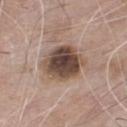The lesion was photographed on a routine skin check and not biopsied; there is no pathology result. Measured at roughly 5 mm in maximum diameter. The patient is a male in their mid- to late 70s. The tile uses white-light illumination. Automated tile analysis of the lesion measured an area of roughly 17 mm², an eccentricity of roughly 0.45, and a symmetry-axis asymmetry near 0.15. The analysis additionally found a border-irregularity index near 1.5/10, a within-lesion color-variation index near 7/10, and a peripheral color-asymmetry measure near 2. A close-up tile cropped from a whole-body skin photograph, about 15 mm across. The lesion is on the chest.